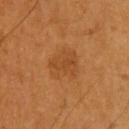On the upper back.
Cropped from a total-body skin-imaging series; the visible field is about 15 mm.
A male patient about 60 years old.
The tile uses cross-polarized illumination.
The recorded lesion diameter is about 4 mm.